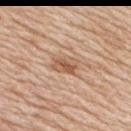Impression:
No biopsy was performed on this lesion — it was imaged during a full skin examination and was not determined to be concerning.
Clinical summary:
Cropped from a whole-body photographic skin survey; the tile spans about 15 mm. Located on the right upper arm. The subject is a male aged approximately 60. About 3 mm across.